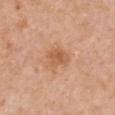body site=the chest; lesion size=~2.5 mm (longest diameter); tile lighting=white-light illumination; subject=female, aged 48 to 52; image=~15 mm crop, total-body skin-cancer survey.